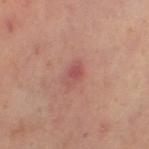- workup · imaged on a skin check; not biopsied
- image source · ~15 mm crop, total-body skin-cancer survey
- illumination · cross-polarized illumination
- automated lesion analysis · a footprint of about 3.5 mm², an outline eccentricity of about 0.85 (0 = round, 1 = elongated), and a shape-asymmetry score of about 0.2 (0 = symmetric); border irregularity of about 2 on a 0–10 scale, internal color variation of about 2 on a 0–10 scale, and radial color variation of about 1; an automated nevus-likeness rating near 0 out of 100
- location · the left thigh
- lesion diameter · about 3 mm
- patient · female, about 40 years old